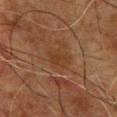biopsy_status: not biopsied; imaged during a skin examination
lesion_size:
  long_diameter_mm_approx: 3.0
image:
  source: total-body photography crop
  field_of_view_mm: 15
patient:
  sex: male
  age_approx: 60
site: chest